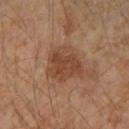Notes:
* follow-up · total-body-photography surveillance lesion; no biopsy
* patient · female, aged around 45
* image source · ~15 mm crop, total-body skin-cancer survey
* body site · the right upper arm
* tile lighting · cross-polarized illumination